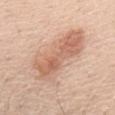Clinical impression: Recorded during total-body skin imaging; not selected for excision or biopsy. Clinical summary: A male patient aged approximately 50. Located on the chest. A 15 mm close-up tile from a total-body photography series done for melanoma screening.